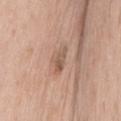follow-up: catalogued during a skin exam; not biopsied | lighting: white-light | subject: male, in their mid-60s | acquisition: ~15 mm crop, total-body skin-cancer survey | automated lesion analysis: an average lesion color of about L≈55 a*≈18 b*≈29 (CIELAB), about 9 CIELAB-L* units darker than the surrounding skin, and a normalized lesion–skin contrast near 6.5 | site: the mid back.